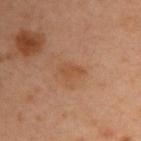Assessment: The lesion was photographed on a routine skin check and not biopsied; there is no pathology result. Image and clinical context: A close-up tile cropped from a whole-body skin photograph, about 15 mm across. On the upper back. A male patient, aged approximately 40. Approximately 2.5 mm at its widest. This is a cross-polarized tile.